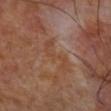No biopsy was performed on this lesion — it was imaged during a full skin examination and was not determined to be concerning.